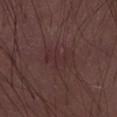notes: catalogued during a skin exam; not biopsied
automated lesion analysis: a within-lesion color-variation index near 2.5/10 and a peripheral color-asymmetry measure near 1
lighting: white-light
image: ~15 mm crop, total-body skin-cancer survey
anatomic site: the abdomen
patient: male, about 80 years old